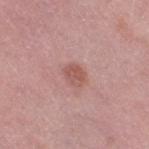Notes:
* automated lesion analysis: an area of roughly 4 mm², an outline eccentricity of about 0.7 (0 = round, 1 = elongated), and two-axis asymmetry of about 0.2; roughly 9 lightness units darker than nearby skin and a normalized border contrast of about 6.5
* location: the leg
* imaging modality: ~15 mm crop, total-body skin-cancer survey
* subject: female, aged 38–42